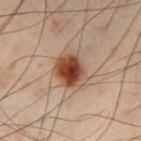{
  "biopsy_status": "not biopsied; imaged during a skin examination",
  "site": "leg",
  "lesion_size": {
    "long_diameter_mm_approx": 5.0
  },
  "image": {
    "source": "total-body photography crop",
    "field_of_view_mm": 15
  },
  "patient": {
    "sex": "male",
    "age_approx": 55
  },
  "lighting": "cross-polarized"
}